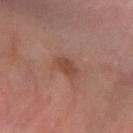Imaged during a routine full-body skin examination; the lesion was not biopsied and no histopathology is available. The lesion is on the right forearm. A male patient, roughly 60 years of age. A lesion tile, about 15 mm wide, cut from a 3D total-body photograph. Measured at roughly 3 mm in maximum diameter.